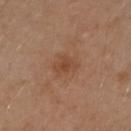Assessment: Imaged during a routine full-body skin examination; the lesion was not biopsied and no histopathology is available. Image and clinical context: The lesion is on the left arm. Captured under cross-polarized illumination. This image is a 15 mm lesion crop taken from a total-body photograph. The lesion's longest dimension is about 3 mm. A female subject, approximately 40 years of age.